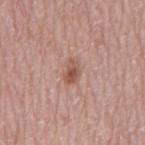follow-up: no biopsy performed (imaged during a skin exam)
diameter: ~2.5 mm (longest diameter)
image source: ~15 mm crop, total-body skin-cancer survey
automated metrics: a lesion color around L≈54 a*≈21 b*≈27 in CIELAB, about 10 CIELAB-L* units darker than the surrounding skin, and a normalized border contrast of about 7.5; a nevus-likeness score of about 75/100
illumination: white-light illumination
patient: male, aged around 70
location: the back